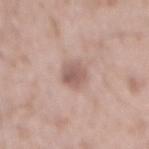• workup · no biopsy performed (imaged during a skin exam)
• illumination · white-light illumination
• subject · male, roughly 50 years of age
• image source · total-body-photography crop, ~15 mm field of view
• body site · the mid back